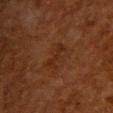Assessment: The lesion was tiled from a total-body skin photograph and was not biopsied.